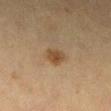Assessment:
The lesion was tiled from a total-body skin photograph and was not biopsied.
Context:
The subject is a female about 40 years old. Imaged with cross-polarized lighting. A 15 mm close-up extracted from a 3D total-body photography capture. About 2.5 mm across. From the left lower leg. Automated image analysis of the tile measured a lesion area of about 5 mm² and two-axis asymmetry of about 0.25. The analysis additionally found an automated nevus-likeness rating near 80 out of 100 and lesion-presence confidence of about 100/100.